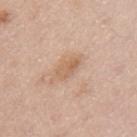image: ~15 mm tile from a whole-body skin photo | location: the arm | lighting: white-light illumination | image-analysis metrics: an area of roughly 4.5 mm², a shape eccentricity near 0.85, and a symmetry-axis asymmetry near 0.25; an average lesion color of about L≈62 a*≈19 b*≈33 (CIELAB), about 8 CIELAB-L* units darker than the surrounding skin, and a normalized border contrast of about 6 | patient: female, approximately 50 years of age | lesion size: ~3 mm (longest diameter).